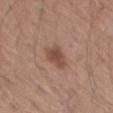Impression:
Imaged during a routine full-body skin examination; the lesion was not biopsied and no histopathology is available.
Image and clinical context:
Measured at roughly 3 mm in maximum diameter. Imaged with white-light lighting. The subject is a male about 65 years old. A 15 mm close-up tile from a total-body photography series done for melanoma screening. The lesion is on the left thigh.